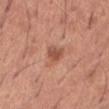Q: Was this lesion biopsied?
A: imaged on a skin check; not biopsied
Q: Patient demographics?
A: male, about 65 years old
Q: How was the tile lit?
A: white-light illumination
Q: What is the anatomic site?
A: the front of the torso
Q: What is the lesion's diameter?
A: about 3 mm
Q: What is the imaging modality?
A: ~15 mm crop, total-body skin-cancer survey